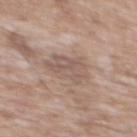No biopsy was performed on this lesion — it was imaged during a full skin examination and was not determined to be concerning. Captured under white-light illumination. The lesion is located on the chest. Cropped from a total-body skin-imaging series; the visible field is about 15 mm. A male subject about 60 years old. Measured at roughly 4.5 mm in maximum diameter.